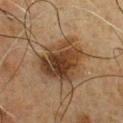Clinical impression: Imaged during a routine full-body skin examination; the lesion was not biopsied and no histopathology is available. Clinical summary: The recorded lesion diameter is about 6 mm. A roughly 15 mm field-of-view crop from a total-body skin photograph. A male subject aged approximately 60. On the chest.